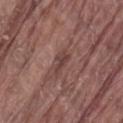Captured during whole-body skin photography for melanoma surveillance; the lesion was not biopsied.
A male patient about 80 years old.
An algorithmic analysis of the crop reported two-axis asymmetry of about 0.4. It also reported a border-irregularity rating of about 4.5/10, a color-variation rating of about 2.5/10, and a peripheral color-asymmetry measure near 1.
Imaged with white-light lighting.
A region of skin cropped from a whole-body photographic capture, roughly 15 mm wide.
From the lower back.
Approximately 3 mm at its widest.